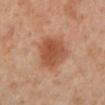TBP lesion metrics: a border-irregularity rating of about 2.5/10, a within-lesion color-variation index near 4/10, and radial color variation of about 1.5 | location: the left lower leg | size: ~5.5 mm (longest diameter) | tile lighting: cross-polarized | patient: female, aged 53–57 | imaging modality: ~15 mm crop, total-body skin-cancer survey.